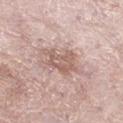| field | value |
|---|---|
| follow-up | imaged on a skin check; not biopsied |
| subject | female, approximately 70 years of age |
| image | ~15 mm tile from a whole-body skin photo |
| site | the left lower leg |